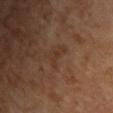notes: catalogued during a skin exam; not biopsied | location: the chest | subject: female, roughly 65 years of age | illumination: cross-polarized illumination | image source: ~15 mm crop, total-body skin-cancer survey.